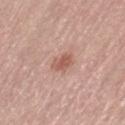Located on the left thigh.
A 15 mm close-up extracted from a 3D total-body photography capture.
The tile uses white-light illumination.
The subject is a female aged 63–67.
Approximately 2.5 mm at its widest.